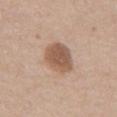Captured during whole-body skin photography for melanoma surveillance; the lesion was not biopsied. Imaged with white-light lighting. Longest diameter approximately 4.5 mm. The lesion-visualizer software estimated a lesion area of about 12 mm², a shape eccentricity near 0.5, and a shape-asymmetry score of about 0.15 (0 = symmetric). It also reported an average lesion color of about L≈56 a*≈18 b*≈29 (CIELAB) and a lesion–skin lightness drop of about 12. It also reported a peripheral color-asymmetry measure near 1. The patient is a male aged approximately 55. From the abdomen. Cropped from a total-body skin-imaging series; the visible field is about 15 mm.